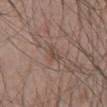follow-up: imaged on a skin check; not biopsied | illumination: white-light illumination | body site: the mid back | size: ≈2.5 mm | acquisition: ~15 mm crop, total-body skin-cancer survey | subject: male, aged 43–47.